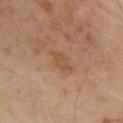follow-up: catalogued during a skin exam; not biopsied | body site: the front of the torso | patient: male, in their mid- to late 60s | diameter: ~3.5 mm (longest diameter) | illumination: cross-polarized | imaging modality: ~15 mm crop, total-body skin-cancer survey | image-analysis metrics: a shape-asymmetry score of about 0.3 (0 = symmetric); an average lesion color of about L≈51 a*≈20 b*≈34 (CIELAB), a lesion–skin lightness drop of about 7, and a normalized lesion–skin contrast near 6; a classifier nevus-likeness of about 0/100 and a detector confidence of about 100 out of 100 that the crop contains a lesion.